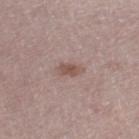Acquisition and patient details:
This is a white-light tile. The lesion is located on the left thigh. Automated image analysis of the tile measured a lesion–skin lightness drop of about 9 and a normalized lesion–skin contrast near 7. The analysis additionally found border irregularity of about 2.5 on a 0–10 scale, a color-variation rating of about 3/10, and a peripheral color-asymmetry measure near 1. It also reported an automated nevus-likeness rating near 30 out of 100. A female subject in their mid- to late 60s. A 15 mm close-up extracted from a 3D total-body photography capture.